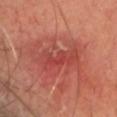The lesion was tiled from a total-body skin photograph and was not biopsied. A 15 mm crop from a total-body photograph taken for skin-cancer surveillance. Imaged with cross-polarized lighting. A male patient, aged approximately 70. Located on the head or neck.